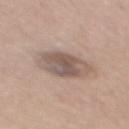This lesion was catalogued during total-body skin photography and was not selected for biopsy.
The lesion's longest dimension is about 5 mm.
Imaged with white-light lighting.
A male subject approximately 60 years of age.
A 15 mm close-up tile from a total-body photography series done for melanoma screening.
From the mid back.